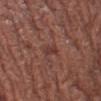Findings:
* workup — total-body-photography surveillance lesion; no biopsy
* subject — female, aged 48 to 52
* site — the left lower leg
* tile lighting — white-light
* acquisition — total-body-photography crop, ~15 mm field of view
* lesion size — ≈2.5 mm